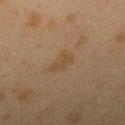{"biopsy_status": "not biopsied; imaged during a skin examination", "image": {"source": "total-body photography crop", "field_of_view_mm": 15}, "patient": {"sex": "female", "age_approx": 40}, "site": "right upper arm"}